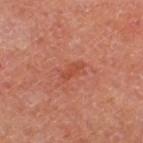Notes:
• biopsy status — no biopsy performed (imaged during a skin exam)
• subject — female
• image source — 15 mm crop, total-body photography
• body site — the left thigh